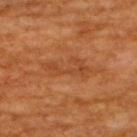No biopsy was performed on this lesion — it was imaged during a full skin examination and was not determined to be concerning.
Cropped from a total-body skin-imaging series; the visible field is about 15 mm.
The lesion-visualizer software estimated a footprint of about 7.5 mm², a shape eccentricity near 0.95, and a symmetry-axis asymmetry near 0.55. The software also gave an automated nevus-likeness rating near 0 out of 100 and a lesion-detection confidence of about 95/100.
From the back.
Captured under cross-polarized illumination.
The lesion's longest dimension is about 5.5 mm.
The subject is a male aged 58 to 62.